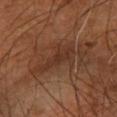biopsy_status: not biopsied; imaged during a skin examination
patient:
  sex: male
  age_approx: 60
site: left forearm
image:
  source: total-body photography crop
  field_of_view_mm: 15
automated_metrics:
  area_mm2_approx: 6.0
  eccentricity: 0.95
  shape_asymmetry: 0.3
  border_irregularity_0_10: 5.0
  color_variation_0_10: 1.5
  peripheral_color_asymmetry: 0.5
lighting: cross-polarized
lesion_size:
  long_diameter_mm_approx: 5.0